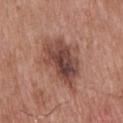| field | value |
|---|---|
| biopsy status | imaged on a skin check; not biopsied |
| imaging modality | ~15 mm crop, total-body skin-cancer survey |
| site | the back |
| tile lighting | white-light |
| automated lesion analysis | a within-lesion color-variation index near 7/10 and radial color variation of about 2; a classifier nevus-likeness of about 10/100 and a detector confidence of about 100 out of 100 that the crop contains a lesion |
| patient | male, aged around 65 |